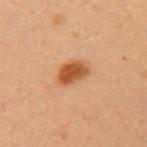Cropped from a whole-body photographic skin survey; the tile spans about 15 mm.
The lesion is located on the left upper arm.
Measured at roughly 3.5 mm in maximum diameter.
The patient is a female in their 40s.